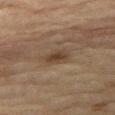Automated tile analysis of the lesion measured a footprint of about 5 mm², an outline eccentricity of about 0.75 (0 = round, 1 = elongated), and a shape-asymmetry score of about 0.3 (0 = symmetric). The software also gave an average lesion color of about L≈35 a*≈14 b*≈25 (CIELAB), a lesion–skin lightness drop of about 8, and a lesion-to-skin contrast of about 7.5 (normalized; higher = more distinct). The software also gave border irregularity of about 2.5 on a 0–10 scale, internal color variation of about 2.5 on a 0–10 scale, and radial color variation of about 1. Cropped from a total-body skin-imaging series; the visible field is about 15 mm. A female patient, aged around 80. The tile uses cross-polarized illumination. About 3 mm across. On the right thigh.